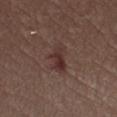biopsy_status: not biopsied; imaged during a skin examination
automated_metrics:
  area_mm2_approx: 6.0
  eccentricity: 0.85
  peripheral_color_asymmetry: 1.5
  nevus_likeness_0_100: 35
  lesion_detection_confidence_0_100: 100
patient:
  sex: female
  age_approx: 30
site: leg
image:
  source: total-body photography crop
  field_of_view_mm: 15
lesion_size:
  long_diameter_mm_approx: 3.5
lighting: white-light